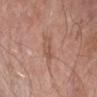No biopsy was performed on this lesion — it was imaged during a full skin examination and was not determined to be concerning.
Automated image analysis of the tile measured an outline eccentricity of about 0.8 (0 = round, 1 = elongated) and a symmetry-axis asymmetry near 0.3. The software also gave a border-irregularity rating of about 3.5/10, internal color variation of about 3.5 on a 0–10 scale, and a peripheral color-asymmetry measure near 1.5.
The subject is a male in their mid- to late 60s.
The lesion's longest dimension is about 3 mm.
The tile uses cross-polarized illumination.
On the left forearm.
A 15 mm close-up tile from a total-body photography series done for melanoma screening.